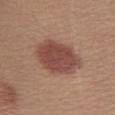Captured during whole-body skin photography for melanoma surveillance; the lesion was not biopsied.
The tile uses white-light illumination.
The recorded lesion diameter is about 6 mm.
A male patient aged 28–32.
Located on the left lower leg.
This image is a 15 mm lesion crop taken from a total-body photograph.